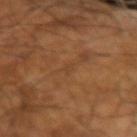The lesion was photographed on a routine skin check and not biopsied; there is no pathology result.
The patient is a male approximately 65 years of age.
A 15 mm crop from a total-body photograph taken for skin-cancer surveillance.
Automated image analysis of the tile measured two-axis asymmetry of about 0.4. It also reported an average lesion color of about L≈39 a*≈19 b*≈32 (CIELAB) and roughly 4 lightness units darker than nearby skin. It also reported a border-irregularity rating of about 3/10 and a within-lesion color-variation index near 0/10.
Imaged with cross-polarized lighting.
The recorded lesion diameter is about 1 mm.